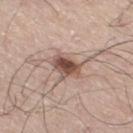| feature | finding |
|---|---|
| follow-up | no biopsy performed (imaged during a skin exam) |
| imaging modality | ~15 mm crop, total-body skin-cancer survey |
| illumination | white-light |
| subject | male, aged 68–72 |
| image-analysis metrics | a footprint of about 7.5 mm² and a shape-asymmetry score of about 0.25 (0 = symmetric); roughly 15 lightness units darker than nearby skin and a normalized lesion–skin contrast near 10; border irregularity of about 3 on a 0–10 scale and a color-variation rating of about 6/10; a nevus-likeness score of about 90/100 |
| lesion size | ≈3.5 mm |
| body site | the left leg |